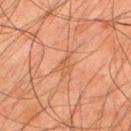Part of a total-body skin-imaging series; this lesion was reviewed on a skin check and was not flagged for biopsy. Captured under cross-polarized illumination. On the back. The total-body-photography lesion software estimated an outline eccentricity of about 0.85 (0 = round, 1 = elongated) and a symmetry-axis asymmetry near 0.45. Cropped from a whole-body photographic skin survey; the tile spans about 15 mm. A male subject aged 43–47. About 3 mm across.